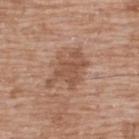Clinical impression: Captured during whole-body skin photography for melanoma surveillance; the lesion was not biopsied. Image and clinical context: On the upper back. The lesion's longest dimension is about 5.5 mm. A lesion tile, about 15 mm wide, cut from a 3D total-body photograph. A male patient, aged 48–52. The total-body-photography lesion software estimated an average lesion color of about L≈52 a*≈20 b*≈30 (CIELAB), about 9 CIELAB-L* units darker than the surrounding skin, and a normalized border contrast of about 6.5. The analysis additionally found a border-irregularity index near 6/10 and peripheral color asymmetry of about 1. And it measured a detector confidence of about 100 out of 100 that the crop contains a lesion.